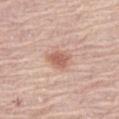Context:
Captured under white-light illumination. Cropped from a whole-body photographic skin survey; the tile spans about 15 mm. On the left thigh. The lesion's longest dimension is about 3 mm. Automated image analysis of the tile measured a lesion color around L≈61 a*≈23 b*≈28 in CIELAB, a lesion–skin lightness drop of about 11, and a normalized border contrast of about 7. And it measured a lesion-detection confidence of about 100/100. A female subject aged around 70.